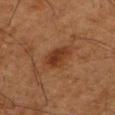<lesion>
<biopsy_status>not biopsied; imaged during a skin examination</biopsy_status>
<lesion_size>
  <long_diameter_mm_approx>3.0</long_diameter_mm_approx>
</lesion_size>
<image>
  <source>total-body photography crop</source>
  <field_of_view_mm>15</field_of_view_mm>
</image>
<patient>
  <sex>male</sex>
  <age_approx>80</age_approx>
</patient>
<lighting>cross-polarized</lighting>
<site>leg</site>
</lesion>